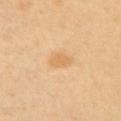  biopsy_status: not biopsied; imaged during a skin examination
  site: right upper arm
  patient:
    sex: female
    age_approx: 50
  lesion_size:
    long_diameter_mm_approx: 3.0
  image:
    source: total-body photography crop
    field_of_view_mm: 15
  lighting: cross-polarized